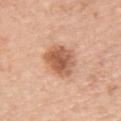Background:
The recorded lesion diameter is about 4 mm. The tile uses white-light illumination. A 15 mm close-up tile from a total-body photography series done for melanoma screening. Located on the upper back. The patient is a female approximately 65 years of age.